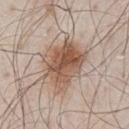{
  "patient": {
    "sex": "male",
    "age_approx": 80
  },
  "lesion_size": {
    "long_diameter_mm_approx": 5.5
  },
  "site": "chest",
  "lighting": "white-light",
  "image": {
    "source": "total-body photography crop",
    "field_of_view_mm": 15
  }
}